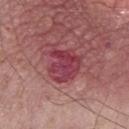{"biopsy_status": "not biopsied; imaged during a skin examination", "automated_metrics": {"area_mm2_approx": 14.0, "eccentricity": 0.5, "shape_asymmetry": 0.35, "cielab_L": 42, "cielab_a": 34, "cielab_b": 17, "vs_skin_darker_L": 9.0, "vs_skin_contrast_norm": 8.0, "border_irregularity_0_10": 4.0, "color_variation_0_10": 5.5, "peripheral_color_asymmetry": 2.0}, "site": "chest", "image": {"source": "total-body photography crop", "field_of_view_mm": 15}, "lesion_size": {"long_diameter_mm_approx": 5.0}, "patient": {"sex": "male", "age_approx": 75}}